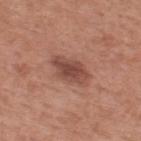The lesion was photographed on a routine skin check and not biopsied; there is no pathology result. The subject is a male aged 53 to 57. The lesion's longest dimension is about 4.5 mm. On the upper back. A 15 mm crop from a total-body photograph taken for skin-cancer surveillance. The lesion-visualizer software estimated a nevus-likeness score of about 55/100 and a lesion-detection confidence of about 100/100. Imaged with white-light lighting.